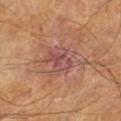| field | value |
|---|---|
| biopsy status | imaged on a skin check; not biopsied |
| patient | male, aged around 60 |
| illumination | cross-polarized illumination |
| automated lesion analysis | a lesion area of about 17 mm², a shape eccentricity near 0.75, and two-axis asymmetry of about 0.35; a lesion color around L≈49 a*≈22 b*≈25 in CIELAB, about 7 CIELAB-L* units darker than the surrounding skin, and a normalized border contrast of about 7 |
| location | the leg |
| imaging modality | 15 mm crop, total-body photography |
| lesion size | ≈6.5 mm |